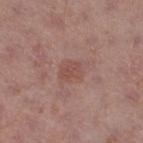Notes:
- workup — catalogued during a skin exam; not biopsied
- image — ~15 mm tile from a whole-body skin photo
- automated lesion analysis — an area of roughly 5 mm², an eccentricity of roughly 0.6, and a symmetry-axis asymmetry near 0.2; a mean CIELAB color near L≈49 a*≈22 b*≈24, a lesion–skin lightness drop of about 7, and a normalized border contrast of about 5.5; a classifier nevus-likeness of about 20/100
- patient — male, approximately 70 years of age
- diameter — ≈2.5 mm
- body site — the right thigh
- tile lighting — white-light